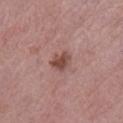biopsy_status: not biopsied; imaged during a skin examination
patient:
  sex: female
  age_approx: 65
site: leg
image:
  source: total-body photography crop
  field_of_view_mm: 15